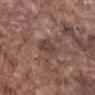Captured during whole-body skin photography for melanoma surveillance; the lesion was not biopsied.
Cropped from a whole-body photographic skin survey; the tile spans about 15 mm.
Longest diameter approximately 3 mm.
An algorithmic analysis of the crop reported an area of roughly 5.5 mm², a shape eccentricity near 0.45, and a symmetry-axis asymmetry near 0.3. The software also gave a lesion color around L≈41 a*≈16 b*≈20 in CIELAB, roughly 8 lightness units darker than nearby skin, and a lesion-to-skin contrast of about 7 (normalized; higher = more distinct). And it measured a border-irregularity rating of about 3/10 and radial color variation of about 1. The analysis additionally found a nevus-likeness score of about 0/100 and a lesion-detection confidence of about 85/100.
A male subject, about 75 years old.
Located on the mid back.